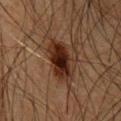<case>
  <biopsy_status>not biopsied; imaged during a skin examination</biopsy_status>
  <site>front of the torso</site>
  <automated_metrics>
    <area_mm2_approx>12.0</area_mm2_approx>
    <eccentricity>0.85</eccentricity>
    <shape_asymmetry>0.2</shape_asymmetry>
    <border_irregularity_0_10>2.5</border_irregularity_0_10>
    <color_variation_0_10>6.0</color_variation_0_10>
    <peripheral_color_asymmetry>1.5</peripheral_color_asymmetry>
    <nevus_likeness_0_100>90</nevus_likeness_0_100>
    <lesion_detection_confidence_0_100>100</lesion_detection_confidence_0_100>
  </automated_metrics>
  <image>
    <source>total-body photography crop</source>
    <field_of_view_mm>15</field_of_view_mm>
  </image>
  <patient>
    <sex>male</sex>
    <age_approx>65</age_approx>
  </patient>
  <lesion_size>
    <long_diameter_mm_approx>5.5</long_diameter_mm_approx>
  </lesion_size>
</case>